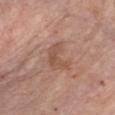A 15 mm close-up tile from a total-body photography series done for melanoma screening.
From the chest.
A female subject in their mid-60s.
The recorded lesion diameter is about 4 mm.
Automated tile analysis of the lesion measured an area of roughly 7 mm², an outline eccentricity of about 0.75 (0 = round, 1 = elongated), and a symmetry-axis asymmetry near 0.55. The analysis additionally found a mean CIELAB color near L≈52 a*≈20 b*≈29, a lesion–skin lightness drop of about 7, and a normalized lesion–skin contrast near 5.5.